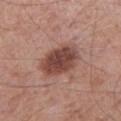A male patient about 55 years old.
This is a white-light tile.
The lesion is located on the leg.
A 15 mm crop from a total-body photograph taken for skin-cancer surveillance.